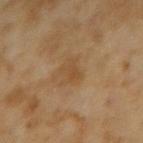Assessment:
No biopsy was performed on this lesion — it was imaged during a full skin examination and was not determined to be concerning.
Acquisition and patient details:
On the left forearm. A female subject aged approximately 60. Measured at roughly 2.5 mm in maximum diameter. A lesion tile, about 15 mm wide, cut from a 3D total-body photograph. This is a cross-polarized tile. An algorithmic analysis of the crop reported a lesion color around L≈43 a*≈16 b*≈33 in CIELAB, roughly 5 lightness units darker than nearby skin, and a normalized border contrast of about 5. The analysis additionally found a border-irregularity index near 3/10, a color-variation rating of about 2/10, and radial color variation of about 1. The analysis additionally found a classifier nevus-likeness of about 0/100 and a lesion-detection confidence of about 100/100.